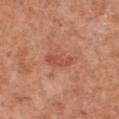Assessment:
Part of a total-body skin-imaging series; this lesion was reviewed on a skin check and was not flagged for biopsy.
Clinical summary:
Longest diameter approximately 4 mm. Located on the chest. Automated tile analysis of the lesion measured a footprint of about 6 mm² and a shape eccentricity near 0.85. The software also gave an average lesion color of about L≈53 a*≈29 b*≈33 (CIELAB), a lesion–skin lightness drop of about 8, and a normalized border contrast of about 5.5. The analysis additionally found a color-variation rating of about 2.5/10. The tile uses white-light illumination. The patient is a female in their mid-30s. Cropped from a whole-body photographic skin survey; the tile spans about 15 mm.